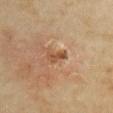Context: A region of skin cropped from a whole-body photographic capture, roughly 15 mm wide. Approximately 3 mm at its widest. A female subject in their mid-30s. The lesion-visualizer software estimated a lesion area of about 3.5 mm² and a symmetry-axis asymmetry near 0.4. The software also gave a mean CIELAB color near L≈49 a*≈21 b*≈34 and about 9 CIELAB-L* units darker than the surrounding skin. The software also gave a border-irregularity index near 4/10, internal color variation of about 1.5 on a 0–10 scale, and peripheral color asymmetry of about 0.5. From the chest. Imaged with cross-polarized lighting.